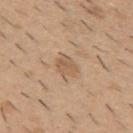workup: imaged on a skin check; not biopsied | patient: male, approximately 40 years of age | anatomic site: the upper back | lesion diameter: ~2.5 mm (longest diameter) | imaging modality: ~15 mm tile from a whole-body skin photo.